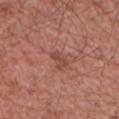The lesion was photographed on a routine skin check and not biopsied; there is no pathology result.
A roughly 15 mm field-of-view crop from a total-body skin photograph.
The lesion is on the arm.
The subject is a male approximately 50 years of age.